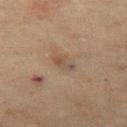notes: catalogued during a skin exam; not biopsied | size: ≈2.5 mm | tile lighting: cross-polarized illumination | anatomic site: the left thigh | patient: female, aged approximately 55 | acquisition: 15 mm crop, total-body photography.